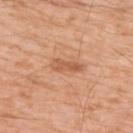Impression:
Recorded during total-body skin imaging; not selected for excision or biopsy.
Image and clinical context:
A male patient aged 58–62. A region of skin cropped from a whole-body photographic capture, roughly 15 mm wide. The lesion is on the upper back.